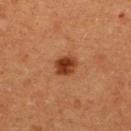| key | value |
|---|---|
| workup | no biopsy performed (imaged during a skin exam) |
| site | the upper back |
| imaging modality | total-body-photography crop, ~15 mm field of view |
| subject | female, aged 38–42 |
| diameter | ~2.5 mm (longest diameter) |
| tile lighting | cross-polarized illumination |
| automated metrics | a footprint of about 5 mm², an outline eccentricity of about 0.45 (0 = round, 1 = elongated), and a shape-asymmetry score of about 0.2 (0 = symmetric); a nevus-likeness score of about 100/100 and a lesion-detection confidence of about 100/100 |